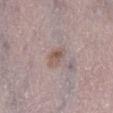biopsy status: total-body-photography surveillance lesion; no biopsy | lighting: white-light | body site: the right lower leg | image source: ~15 mm crop, total-body skin-cancer survey | image-analysis metrics: a border-irregularity rating of about 2/10; an automated nevus-likeness rating near 60 out of 100 and a detector confidence of about 100 out of 100 that the crop contains a lesion | patient: male, aged 68 to 72.